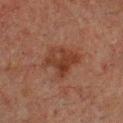This lesion was catalogued during total-body skin photography and was not selected for biopsy.